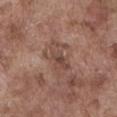follow-up = total-body-photography surveillance lesion; no biopsy
subject = male, in their mid- to late 70s
tile lighting = white-light
image source = ~15 mm crop, total-body skin-cancer survey
site = the front of the torso
lesion diameter = ~4.5 mm (longest diameter)